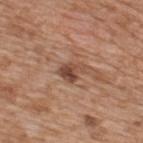The lesion was photographed on a routine skin check and not biopsied; there is no pathology result.
A close-up tile cropped from a whole-body skin photograph, about 15 mm across.
Located on the upper back.
A male subject, in their mid-60s.